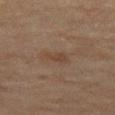Assessment:
Imaged during a routine full-body skin examination; the lesion was not biopsied and no histopathology is available.
Clinical summary:
Located on the leg. A female patient, aged around 60. A 15 mm crop from a total-body photograph taken for skin-cancer surveillance. Longest diameter approximately 2.5 mm. Captured under cross-polarized illumination.